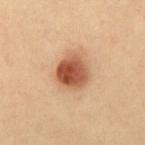| key | value |
|---|---|
| workup | imaged on a skin check; not biopsied |
| lesion size | ≈3.5 mm |
| illumination | cross-polarized illumination |
| body site | the back |
| acquisition | ~15 mm tile from a whole-body skin photo |
| subject | male, roughly 40 years of age |